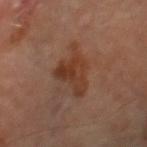workup: no biopsy performed (imaged during a skin exam); patient: male, aged approximately 70; image source: 15 mm crop, total-body photography; lesion diameter: ≈5.5 mm; illumination: cross-polarized; site: the right thigh.